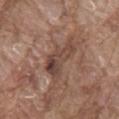Part of a total-body skin-imaging series; this lesion was reviewed on a skin check and was not flagged for biopsy.
A 15 mm close-up tile from a total-body photography series done for melanoma screening.
From the mid back.
An algorithmic analysis of the crop reported a footprint of about 11 mm² and an eccentricity of roughly 0.9. And it measured a mean CIELAB color near L≈44 a*≈18 b*≈24 and a normalized lesion–skin contrast near 7. And it measured a classifier nevus-likeness of about 0/100 and a lesion-detection confidence of about 65/100.
The recorded lesion diameter is about 5.5 mm.
The subject is a male aged 78–82.